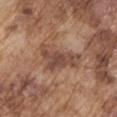workup — no biopsy performed (imaged during a skin exam) | automated metrics — a lesion area of about 12 mm², an outline eccentricity of about 0.85 (0 = round, 1 = elongated), and a symmetry-axis asymmetry near 0.45; about 9 CIELAB-L* units darker than the surrounding skin; border irregularity of about 5 on a 0–10 scale, a color-variation rating of about 4/10, and peripheral color asymmetry of about 1; a lesion-detection confidence of about 85/100 | imaging modality — total-body-photography crop, ~15 mm field of view | subject — male, aged around 75 | body site — the right upper arm | tile lighting — white-light illumination.